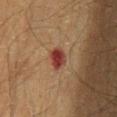About 2.5 mm across. This image is a 15 mm lesion crop taken from a total-body photograph. From the lower back. A male subject aged around 65. This is a cross-polarized tile.